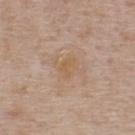workup = total-body-photography surveillance lesion; no biopsy | lesion size = ~2.5 mm (longest diameter) | tile lighting = white-light illumination | imaging modality = ~15 mm crop, total-body skin-cancer survey | location = the back | patient = male, about 75 years old.